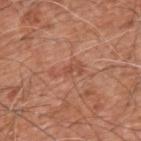Assessment:
The lesion was tiled from a total-body skin photograph and was not biopsied.
Acquisition and patient details:
The lesion is located on the upper back. Captured under white-light illumination. Approximately 4 mm at its widest. A male patient, about 60 years old. Automated image analysis of the tile measured border irregularity of about 6.5 on a 0–10 scale and internal color variation of about 2 on a 0–10 scale. And it measured a classifier nevus-likeness of about 0/100. A 15 mm close-up extracted from a 3D total-body photography capture.